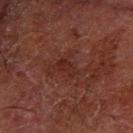Impression: The lesion was tiled from a total-body skin photograph and was not biopsied. Image and clinical context: Longest diameter approximately 2.5 mm. The lesion-visualizer software estimated border irregularity of about 4.5 on a 0–10 scale. The analysis additionally found an automated nevus-likeness rating near 0 out of 100 and lesion-presence confidence of about 100/100. A male subject, in their 70s. A 15 mm close-up extracted from a 3D total-body photography capture. The lesion is on the arm. Captured under cross-polarized illumination.